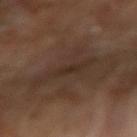Q: Was this lesion biopsied?
A: total-body-photography surveillance lesion; no biopsy
Q: What is the lesion's diameter?
A: ~5.5 mm (longest diameter)
Q: What are the patient's age and sex?
A: male, roughly 65 years of age
Q: What is the anatomic site?
A: the right forearm
Q: What lighting was used for the tile?
A: cross-polarized illumination
Q: What is the imaging modality?
A: total-body-photography crop, ~15 mm field of view